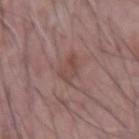Case summary:
– body site: the right forearm
– imaging modality: total-body-photography crop, ~15 mm field of view
– automated metrics: an average lesion color of about L≈46 a*≈19 b*≈22 (CIELAB), a lesion–skin lightness drop of about 7, and a lesion-to-skin contrast of about 5.5 (normalized; higher = more distinct); a border-irregularity rating of about 4/10, a color-variation rating of about 3.5/10, and a peripheral color-asymmetry measure near 1; a nevus-likeness score of about 0/100 and a detector confidence of about 100 out of 100 that the crop contains a lesion
– patient: male, aged approximately 70
– size: about 2.5 mm
– lighting: white-light